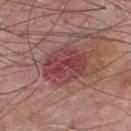{
  "biopsy_status": "not biopsied; imaged during a skin examination"
}